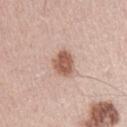workup=no biopsy performed (imaged during a skin exam).